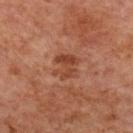Assessment:
The lesion was photographed on a routine skin check and not biopsied; there is no pathology result.
Clinical summary:
About 3 mm across. The subject is a female aged approximately 60. The lesion-visualizer software estimated an eccentricity of roughly 0.45 and a symmetry-axis asymmetry near 0.25. It also reported a classifier nevus-likeness of about 0/100 and a detector confidence of about 100 out of 100 that the crop contains a lesion. Located on the back. A close-up tile cropped from a whole-body skin photograph, about 15 mm across.